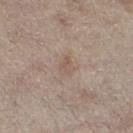lesion size: ~2.5 mm (longest diameter) | patient: male, about 70 years old | site: the leg | lighting: white-light | image-analysis metrics: an area of roughly 4.5 mm², a shape eccentricity near 0.65, and two-axis asymmetry of about 0.3; an average lesion color of about L≈56 a*≈14 b*≈24 (CIELAB), about 6 CIELAB-L* units darker than the surrounding skin, and a lesion-to-skin contrast of about 4.5 (normalized; higher = more distinct); a border-irregularity rating of about 2.5/10, internal color variation of about 2 on a 0–10 scale, and peripheral color asymmetry of about 0.5; a detector confidence of about 100 out of 100 that the crop contains a lesion | acquisition: ~15 mm tile from a whole-body skin photo.